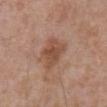Imaged during a routine full-body skin examination; the lesion was not biopsied and no histopathology is available.
Measured at roughly 4 mm in maximum diameter.
The subject is a male in their 70s.
Automated image analysis of the tile measured a border-irregularity index near 3/10, internal color variation of about 3.5 on a 0–10 scale, and radial color variation of about 1. And it measured an automated nevus-likeness rating near 0 out of 100 and lesion-presence confidence of about 100/100.
On the abdomen.
A region of skin cropped from a whole-body photographic capture, roughly 15 mm wide.